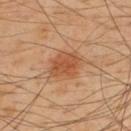Q: Is there a histopathology result?
A: catalogued during a skin exam; not biopsied
Q: Who is the patient?
A: male, aged approximately 50
Q: How was this image acquired?
A: ~15 mm crop, total-body skin-cancer survey
Q: Where on the body is the lesion?
A: the upper back
Q: How was the tile lit?
A: cross-polarized illumination
Q: Automated lesion metrics?
A: a footprint of about 10 mm², a shape eccentricity near 0.6, and a shape-asymmetry score of about 0.2 (0 = symmetric); a lesion–skin lightness drop of about 9 and a normalized border contrast of about 7; border irregularity of about 2.5 on a 0–10 scale and peripheral color asymmetry of about 1; a classifier nevus-likeness of about 95/100 and a detector confidence of about 100 out of 100 that the crop contains a lesion